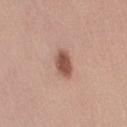biopsy_status: not biopsied; imaged during a skin examination
lesion_size:
  long_diameter_mm_approx: 3.5
image:
  source: total-body photography crop
  field_of_view_mm: 15
lighting: white-light
site: leg
automated_metrics:
  area_mm2_approx: 6.0
  eccentricity: 0.8
  shape_asymmetry: 0.25
  border_irregularity_0_10: 2.0
  color_variation_0_10: 3.0
  peripheral_color_asymmetry: 1.0
  lesion_detection_confidence_0_100: 100
patient:
  sex: female
  age_approx: 35